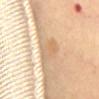Recorded during total-body skin imaging; not selected for excision or biopsy.
Captured under cross-polarized illumination.
The patient is a female roughly 60 years of age.
An algorithmic analysis of the crop reported a footprint of about 5 mm² and an eccentricity of roughly 0.9.
The lesion is located on the chest.
A region of skin cropped from a whole-body photographic capture, roughly 15 mm wide.